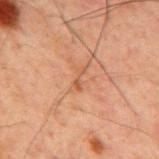workup: imaged on a skin check; not biopsied | lighting: cross-polarized illumination | image-analysis metrics: a shape eccentricity near 0.95 and a shape-asymmetry score of about 0.35 (0 = symmetric); an average lesion color of about L≈43 a*≈20 b*≈29 (CIELAB), about 6 CIELAB-L* units darker than the surrounding skin, and a normalized lesion–skin contrast near 5.5; a nevus-likeness score of about 0/100 and lesion-presence confidence of about 100/100 | patient: male, aged around 60 | anatomic site: the mid back | imaging modality: 15 mm crop, total-body photography | diameter: ~2.5 mm (longest diameter).